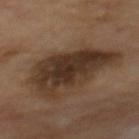– biopsy status · no biopsy performed (imaged during a skin exam)
– anatomic site · the mid back
– subject · male, aged 68–72
– automated metrics · a lesion area of about 30 mm², a shape eccentricity near 0.85, and a symmetry-axis asymmetry near 0.3; a mean CIELAB color near L≈31 a*≈14 b*≈24 and a normalized lesion–skin contrast near 10.5; lesion-presence confidence of about 100/100
– tile lighting · cross-polarized illumination
– image · 15 mm crop, total-body photography